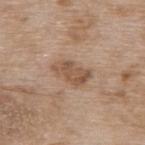Assessment: This lesion was catalogued during total-body skin photography and was not selected for biopsy. Acquisition and patient details: From the upper back. This is a white-light tile. A female patient aged approximately 75. A 15 mm close-up extracted from a 3D total-body photography capture. The lesion's longest dimension is about 4.5 mm.